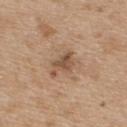Assessment: This lesion was catalogued during total-body skin photography and was not selected for biopsy. Clinical summary: A female patient, in their mid-40s. Imaged with white-light lighting. The total-body-photography lesion software estimated a lesion area of about 5 mm² and a symmetry-axis asymmetry near 0.4. And it measured a mean CIELAB color near L≈51 a*≈19 b*≈31 and a normalized lesion–skin contrast near 7.5. The analysis additionally found a nevus-likeness score of about 30/100 and a detector confidence of about 100 out of 100 that the crop contains a lesion. About 3.5 mm across. The lesion is located on the upper back. A 15 mm close-up tile from a total-body photography series done for melanoma screening.